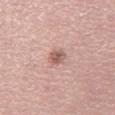Context: An algorithmic analysis of the crop reported an area of roughly 4 mm², a shape eccentricity near 0.6, and a shape-asymmetry score of about 0.2 (0 = symmetric). The analysis additionally found a border-irregularity rating of about 2/10, a within-lesion color-variation index near 3/10, and radial color variation of about 1. The software also gave an automated nevus-likeness rating near 50 out of 100 and a lesion-detection confidence of about 100/100. The tile uses white-light illumination. A region of skin cropped from a whole-body photographic capture, roughly 15 mm wide. A female subject, approximately 65 years of age. On the left thigh.